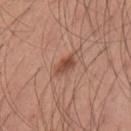The lesion was tiled from a total-body skin photograph and was not biopsied. A male subject, aged 33–37. From the left upper arm. The tile uses white-light illumination. A 15 mm close-up tile from a total-body photography series done for melanoma screening.